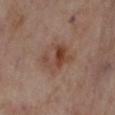Clinical impression:
Captured during whole-body skin photography for melanoma surveillance; the lesion was not biopsied.
Acquisition and patient details:
A female subject, about 55 years old. The total-body-photography lesion software estimated an average lesion color of about L≈44 a*≈20 b*≈27 (CIELAB) and a lesion–skin lightness drop of about 9. The analysis additionally found a border-irregularity rating of about 5/10, a color-variation rating of about 7/10, and a peripheral color-asymmetry measure near 2.5. And it measured an automated nevus-likeness rating near 55 out of 100 and a detector confidence of about 100 out of 100 that the crop contains a lesion. On the left lower leg. A 15 mm crop from a total-body photograph taken for skin-cancer surveillance. Imaged with cross-polarized lighting.